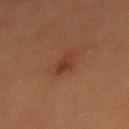Impression:
This lesion was catalogued during total-body skin photography and was not selected for biopsy.
Clinical summary:
A male patient about 40 years old. A 15 mm close-up extracted from a 3D total-body photography capture. About 2.5 mm across. The lesion is on the back.